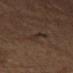This lesion was catalogued during total-body skin photography and was not selected for biopsy. A male patient, about 70 years old. A 15 mm close-up tile from a total-body photography series done for melanoma screening. On the leg.